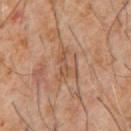biopsy status = total-body-photography surveillance lesion; no biopsy | location = the chest | lighting = cross-polarized | imaging modality = ~15 mm crop, total-body skin-cancer survey | subject = male, aged around 60.